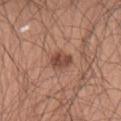workup: imaged on a skin check; not biopsied.